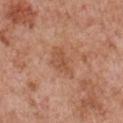follow-up = total-body-photography surveillance lesion; no biopsy | image source = ~15 mm crop, total-body skin-cancer survey | illumination = white-light illumination | subject = male, about 65 years old | automated metrics = a nevus-likeness score of about 0/100 and a lesion-detection confidence of about 100/100 | lesion diameter = ≈4 mm | body site = the chest.